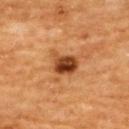Assessment: This lesion was catalogued during total-body skin photography and was not selected for biopsy. Context: Located on the upper back. The lesion-visualizer software estimated an area of roughly 7 mm² and a symmetry-axis asymmetry near 0.2. The analysis additionally found about 15 CIELAB-L* units darker than the surrounding skin and a normalized lesion–skin contrast near 11.5. It also reported a border-irregularity index near 2/10, internal color variation of about 8 on a 0–10 scale, and peripheral color asymmetry of about 3. A male subject aged around 60. Approximately 3.5 mm at its widest. The tile uses cross-polarized illumination. This image is a 15 mm lesion crop taken from a total-body photograph.